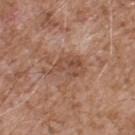Clinical impression: Imaged during a routine full-body skin examination; the lesion was not biopsied and no histopathology is available. Clinical summary: On the upper back. A roughly 15 mm field-of-view crop from a total-body skin photograph. A male patient, about 55 years old. Imaged with white-light lighting. An algorithmic analysis of the crop reported a lesion color around L≈49 a*≈21 b*≈29 in CIELAB, roughly 8 lightness units darker than nearby skin, and a normalized lesion–skin contrast near 6.